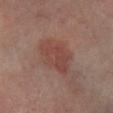Clinical impression: The lesion was tiled from a total-body skin photograph and was not biopsied. Image and clinical context: This is a cross-polarized tile. A 15 mm close-up extracted from a 3D total-body photography capture. A male patient, aged 63 to 67. The lesion is on the right lower leg. Longest diameter approximately 5.5 mm. The total-body-photography lesion software estimated a footprint of about 14 mm², an eccentricity of roughly 0.8, and a symmetry-axis asymmetry near 0.25.